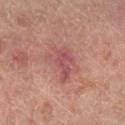follow-up: no biopsy performed (imaged during a skin exam); patient: female, aged approximately 60; anatomic site: the right lower leg; tile lighting: cross-polarized illumination; lesion diameter: ≈4.5 mm; imaging modality: ~15 mm tile from a whole-body skin photo.